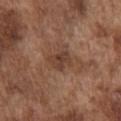notes: total-body-photography surveillance lesion; no biopsy | illumination: white-light | lesion diameter: ~3 mm (longest diameter) | anatomic site: the front of the torso | image source: ~15 mm crop, total-body skin-cancer survey | subject: male, in their mid- to late 70s.